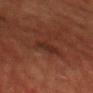The lesion was photographed on a routine skin check and not biopsied; there is no pathology result. The tile uses cross-polarized illumination. A male subject aged approximately 50. A lesion tile, about 15 mm wide, cut from a 3D total-body photograph. From the head or neck. About 2.5 mm across.